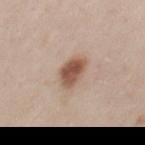{"biopsy_status": "not biopsied; imaged during a skin examination", "site": "chest", "patient": {"sex": "female", "age_approx": 45}, "image": {"source": "total-body photography crop", "field_of_view_mm": 15}}